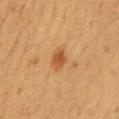Impression:
This lesion was catalogued during total-body skin photography and was not selected for biopsy.
Context:
A 15 mm close-up tile from a total-body photography series done for melanoma screening. A female patient aged 48–52. The lesion is located on the back. Captured under cross-polarized illumination.